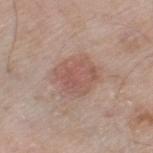Findings:
• patient — male, aged 78 to 82
• anatomic site — the leg
• TBP lesion metrics — an area of roughly 13 mm² and a shape-asymmetry score of about 0.2 (0 = symmetric); a border-irregularity rating of about 2/10, a color-variation rating of about 3/10, and radial color variation of about 1
• image — 15 mm crop, total-body photography
• illumination — white-light